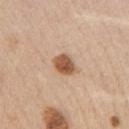Imaged during a routine full-body skin examination; the lesion was not biopsied and no histopathology is available.
From the chest.
A female patient, roughly 70 years of age.
Cropped from a total-body skin-imaging series; the visible field is about 15 mm.